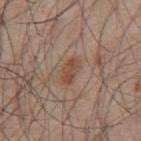Captured during whole-body skin photography for melanoma surveillance; the lesion was not biopsied.
The lesion-visualizer software estimated a footprint of about 4.5 mm², a shape eccentricity near 0.85, and two-axis asymmetry of about 0.25. The software also gave border irregularity of about 2.5 on a 0–10 scale and radial color variation of about 1. The software also gave an automated nevus-likeness rating near 5 out of 100.
From the mid back.
The patient is a male approximately 55 years of age.
Cropped from a whole-body photographic skin survey; the tile spans about 15 mm.
This is a white-light tile.